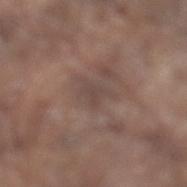This lesion was catalogued during total-body skin photography and was not selected for biopsy.
Cropped from a whole-body photographic skin survey; the tile spans about 15 mm.
On the left lower leg.
The patient is a male aged around 80.